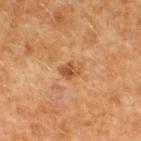Recorded during total-body skin imaging; not selected for excision or biopsy. The lesion is on the upper back. About 2.5 mm across. The subject is a male about 50 years old. A roughly 15 mm field-of-view crop from a total-body skin photograph.